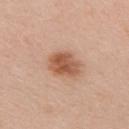Part of a total-body skin-imaging series; this lesion was reviewed on a skin check and was not flagged for biopsy. From the right upper arm. The tile uses white-light illumination. A 15 mm close-up extracted from a 3D total-body photography capture. Measured at roughly 4 mm in maximum diameter. The subject is a female aged around 35.